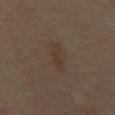| field | value |
|---|---|
| workup | no biopsy performed (imaged during a skin exam) |
| anatomic site | the chest |
| TBP lesion metrics | an area of roughly 3.5 mm², an eccentricity of roughly 0.95, and two-axis asymmetry of about 0.2; a border-irregularity rating of about 4/10, a within-lesion color-variation index near 0/10, and a peripheral color-asymmetry measure near 0; a nevus-likeness score of about 5/100 and a detector confidence of about 100 out of 100 that the crop contains a lesion |
| imaging modality | ~15 mm crop, total-body skin-cancer survey |
| patient | male, in their mid- to late 60s |
| tile lighting | cross-polarized illumination |